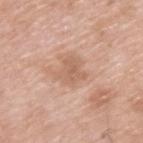Assessment: No biopsy was performed on this lesion — it was imaged during a full skin examination and was not determined to be concerning.